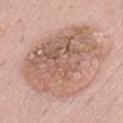This lesion was catalogued during total-body skin photography and was not selected for biopsy. A roughly 15 mm field-of-view crop from a total-body skin photograph. A male subject, about 60 years old. From the mid back. This is a white-light tile. The lesion's longest dimension is about 10.5 mm.